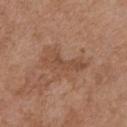lesion diameter: ~5 mm (longest diameter)
patient: female, in their mid- to late 70s
image source: ~15 mm tile from a whole-body skin photo
lighting: white-light
site: the chest